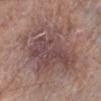Impression:
Imaged during a routine full-body skin examination; the lesion was not biopsied and no histopathology is available.
Clinical summary:
A lesion tile, about 15 mm wide, cut from a 3D total-body photograph. A male patient roughly 55 years of age. The lesion is located on the leg.